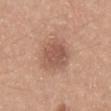biopsy_status: not biopsied; imaged during a skin examination
lesion_size:
  long_diameter_mm_approx: 4.5
image:
  source: total-body photography crop
  field_of_view_mm: 15
site: abdomen
patient:
  sex: male
  age_approx: 20
automated_metrics:
  area_mm2_approx: 13.0
  eccentricity: 0.55
  shape_asymmetry: 0.2
  color_variation_0_10: 3.0
  peripheral_color_asymmetry: 1.0
  nevus_likeness_0_100: 45